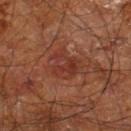Q: Was a biopsy performed?
A: no biopsy performed (imaged during a skin exam)
Q: How large is the lesion?
A: about 4 mm
Q: Who is the patient?
A: male, aged approximately 60
Q: Lesion location?
A: the leg
Q: What did automated image analysis measure?
A: an average lesion color of about L≈34 a*≈26 b*≈28 (CIELAB) and roughly 6 lightness units darker than nearby skin; border irregularity of about 4 on a 0–10 scale, a within-lesion color-variation index near 4.5/10, and radial color variation of about 1.5; a classifier nevus-likeness of about 0/100 and lesion-presence confidence of about 100/100
Q: Illumination type?
A: cross-polarized illumination
Q: What kind of image is this?
A: ~15 mm tile from a whole-body skin photo